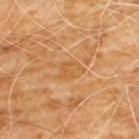Notes:
– notes · catalogued during a skin exam; not biopsied
– image source · ~15 mm crop, total-body skin-cancer survey
– size · ≈2.5 mm
– lighting · cross-polarized
– subject · male, in their 60s
– location · the chest
– TBP lesion metrics · an area of roughly 4 mm², an eccentricity of roughly 0.7, and two-axis asymmetry of about 0.35; an average lesion color of about L≈55 a*≈23 b*≈42 (CIELAB) and a lesion-to-skin contrast of about 4.5 (normalized; higher = more distinct); border irregularity of about 3.5 on a 0–10 scale, internal color variation of about 2 on a 0–10 scale, and a peripheral color-asymmetry measure near 1